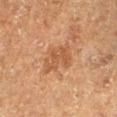biopsy status — catalogued during a skin exam; not biopsied | acquisition — ~15 mm tile from a whole-body skin photo | subject — male, aged around 75 | diameter — about 3.5 mm | site — the right lower leg | image-analysis metrics — an area of roughly 6 mm², a shape eccentricity near 0.65, and a shape-asymmetry score of about 0.5 (0 = symmetric); border irregularity of about 7.5 on a 0–10 scale and a within-lesion color-variation index near 2/10; a nevus-likeness score of about 0/100.